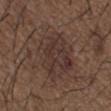Notes:
- workup: total-body-photography surveillance lesion; no biopsy
- lesion diameter: about 6 mm
- site: the upper back
- subject: male, in their 50s
- illumination: white-light illumination
- image source: total-body-photography crop, ~15 mm field of view
- automated metrics: a lesion color around L≈34 a*≈15 b*≈20 in CIELAB, a lesion–skin lightness drop of about 6, and a normalized lesion–skin contrast near 6.5; a classifier nevus-likeness of about 10/100 and lesion-presence confidence of about 100/100